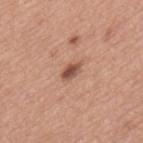Impression: Recorded during total-body skin imaging; not selected for excision or biopsy. Clinical summary: The patient is a male aged approximately 75. Captured under white-light illumination. Measured at roughly 3 mm in maximum diameter. The lesion is on the upper back. Automated image analysis of the tile measured a shape eccentricity near 0.85 and a shape-asymmetry score of about 0.35 (0 = symmetric). A 15 mm close-up extracted from a 3D total-body photography capture.